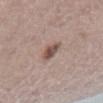A 15 mm crop from a total-body photograph taken for skin-cancer surveillance.
Located on the right lower leg.
Captured under white-light illumination.
Approximately 2.5 mm at its widest.
Automated image analysis of the tile measured an eccentricity of roughly 0.65 and two-axis asymmetry of about 0.25. And it measured internal color variation of about 4.5 on a 0–10 scale and peripheral color asymmetry of about 1.5. The analysis additionally found an automated nevus-likeness rating near 85 out of 100.
The subject is a female about 65 years old.